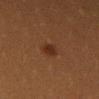Case summary:
– follow-up · no biopsy performed (imaged during a skin exam)
– tile lighting · cross-polarized illumination
– lesion size · ≈2.5 mm
– patient · female, aged 38–42
– acquisition · total-body-photography crop, ~15 mm field of view
– automated metrics · a mean CIELAB color near L≈23 a*≈18 b*≈25 and about 6 CIELAB-L* units darker than the surrounding skin
– body site · the left thigh